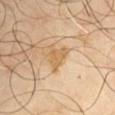workup: no biopsy performed (imaged during a skin exam)
image source: total-body-photography crop, ~15 mm field of view
lesion size: about 2.5 mm
subject: male, about 65 years old
body site: the left upper arm
automated metrics: a mean CIELAB color near L≈59 a*≈17 b*≈38, a lesion–skin lightness drop of about 8, and a normalized border contrast of about 6.5; radial color variation of about 0.5